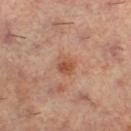Q: Was a biopsy performed?
A: imaged on a skin check; not biopsied
Q: What are the patient's age and sex?
A: female, in their 60s
Q: Automated lesion metrics?
A: a normalized lesion–skin contrast near 7
Q: What is the anatomic site?
A: the right lower leg
Q: What is the imaging modality?
A: ~15 mm crop, total-body skin-cancer survey
Q: How was the tile lit?
A: cross-polarized